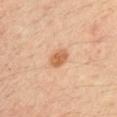size=~3 mm (longest diameter)
tile lighting=cross-polarized illumination
location=the right upper arm
image-analysis metrics=a border-irregularity rating of about 1.5/10, internal color variation of about 2 on a 0–10 scale, and radial color variation of about 0.5; a nevus-likeness score of about 90/100 and lesion-presence confidence of about 100/100
patient=male, aged around 35
image=15 mm crop, total-body photography